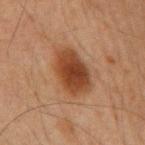Imaged during a routine full-body skin examination; the lesion was not biopsied and no histopathology is available. Imaged with cross-polarized lighting. A male patient aged 63 to 67. The lesion-visualizer software estimated radial color variation of about 1. And it measured a classifier nevus-likeness of about 95/100 and lesion-presence confidence of about 100/100. The lesion is on the back. A 15 mm close-up tile from a total-body photography series done for melanoma screening.